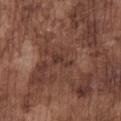Assessment: Imaged during a routine full-body skin examination; the lesion was not biopsied and no histopathology is available. Image and clinical context: A region of skin cropped from a whole-body photographic capture, roughly 15 mm wide. The lesion's longest dimension is about 2.5 mm. A male subject, aged around 75. Automated image analysis of the tile measured about 6 CIELAB-L* units darker than the surrounding skin and a lesion-to-skin contrast of about 6.5 (normalized; higher = more distinct). The analysis additionally found an automated nevus-likeness rating near 0 out of 100. This is a white-light tile. The lesion is located on the chest.